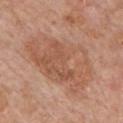Assessment: The lesion was tiled from a total-body skin photograph and was not biopsied.